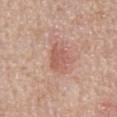Clinical impression: Part of a total-body skin-imaging series; this lesion was reviewed on a skin check and was not flagged for biopsy. Acquisition and patient details: The lesion is located on the abdomen. A male subject aged 63–67. Measured at roughly 4 mm in maximum diameter. A 15 mm close-up extracted from a 3D total-body photography capture. Imaged with white-light lighting.